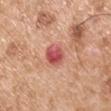workup = imaged on a skin check; not biopsied | diameter = about 3.5 mm | imaging modality = 15 mm crop, total-body photography | tile lighting = white-light illumination | image-analysis metrics = a border-irregularity rating of about 2/10 and a within-lesion color-variation index near 7/10; a classifier nevus-likeness of about 0/100 and lesion-presence confidence of about 100/100 | subject = male, aged 53–57 | anatomic site = the left upper arm.